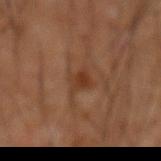biopsy_status: not biopsied; imaged during a skin examination
patient:
  sex: male
  age_approx: 60
lighting: cross-polarized
lesion_size:
  long_diameter_mm_approx: 2.5
image:
  source: total-body photography crop
  field_of_view_mm: 15
site: mid back
automated_metrics:
  cielab_L: 33
  cielab_a: 19
  cielab_b: 28
  vs_skin_darker_L: 7.0
  vs_skin_contrast_norm: 6.5
  border_irregularity_0_10: 3.0
  peripheral_color_asymmetry: 0.5